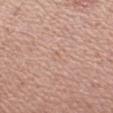The lesion was photographed on a routine skin check and not biopsied; there is no pathology result. Approximately 1.5 mm at its widest. The lesion is on the left forearm. A male patient aged 43 to 47. Cropped from a whole-body photographic skin survey; the tile spans about 15 mm. Imaged with white-light lighting.